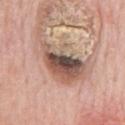The lesion was tiled from a total-body skin photograph and was not biopsied. Captured under white-light illumination. The lesion is on the chest. The patient is a male approximately 80 years of age. A roughly 15 mm field-of-view crop from a total-body skin photograph.